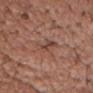Q: Is there a histopathology result?
A: no biopsy performed (imaged during a skin exam)
Q: Who is the patient?
A: male, aged 63 to 67
Q: What kind of image is this?
A: ~15 mm tile from a whole-body skin photo
Q: Where on the body is the lesion?
A: the head or neck